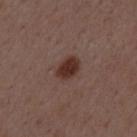* follow-up: no biopsy performed (imaged during a skin exam)
* location: the mid back
* TBP lesion metrics: an average lesion color of about L≈32 a*≈19 b*≈23 (CIELAB) and a lesion–skin lightness drop of about 11; a border-irregularity rating of about 1.5/10 and peripheral color asymmetry of about 1
* image source: 15 mm crop, total-body photography
* subject: male, roughly 50 years of age
* size: ~3 mm (longest diameter)